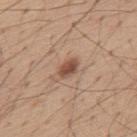subject: male, roughly 55 years of age
image source: total-body-photography crop, ~15 mm field of view
anatomic site: the mid back
tile lighting: white-light
automated metrics: an outline eccentricity of about 0.8 (0 = round, 1 = elongated) and a symmetry-axis asymmetry near 0.2
lesion size: ~3 mm (longest diameter)